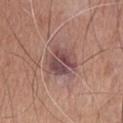Part of a total-body skin-imaging series; this lesion was reviewed on a skin check and was not flagged for biopsy.
The total-body-photography lesion software estimated an average lesion color of about L≈48 a*≈21 b*≈19 (CIELAB) and a lesion–skin lightness drop of about 10.
The lesion is on the head or neck.
Imaged with white-light lighting.
Cropped from a total-body skin-imaging series; the visible field is about 15 mm.
A male patient, aged 43–47.
Measured at roughly 4 mm in maximum diameter.